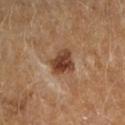Part of a total-body skin-imaging series; this lesion was reviewed on a skin check and was not flagged for biopsy. Measured at roughly 3.5 mm in maximum diameter. Captured under cross-polarized illumination. The subject is a female aged 58–62. A region of skin cropped from a whole-body photographic capture, roughly 15 mm wide. The lesion is on the right forearm.